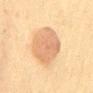follow-up=no biopsy performed (imaged during a skin exam)
patient=female, aged 38 to 42
image=~15 mm tile from a whole-body skin photo
site=the mid back